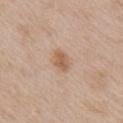Clinical summary:
A region of skin cropped from a whole-body photographic capture, roughly 15 mm wide. The lesion is on the right upper arm. A female subject, aged 38 to 42.